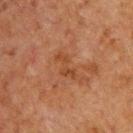Findings:
- biopsy status — catalogued during a skin exam; not biopsied
- lighting — cross-polarized illumination
- imaging modality — 15 mm crop, total-body photography
- TBP lesion metrics — a lesion area of about 5 mm², an outline eccentricity of about 0.9 (0 = round, 1 = elongated), and a shape-asymmetry score of about 0.5 (0 = symmetric); an average lesion color of about L≈34 a*≈19 b*≈29 (CIELAB), about 5 CIELAB-L* units darker than the surrounding skin, and a normalized border contrast of about 6; a border-irregularity index near 6.5/10, a color-variation rating of about 1.5/10, and radial color variation of about 0.5
- size — ≈4 mm
- subject — male, approximately 60 years of age
- body site — the back